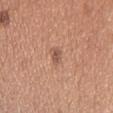Recorded during total-body skin imaging; not selected for excision or biopsy. Cropped from a total-body skin-imaging series; the visible field is about 15 mm. The lesion is on the head or neck. The total-body-photography lesion software estimated a lesion-to-skin contrast of about 6.5 (normalized; higher = more distinct). And it measured a border-irregularity rating of about 3.5/10 and radial color variation of about 0. The analysis additionally found a nevus-likeness score of about 0/100 and lesion-presence confidence of about 100/100. A male patient, aged 28 to 32. The lesion's longest dimension is about 2.5 mm. This is a white-light tile.